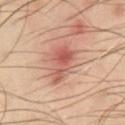lighting: cross-polarized
image: total-body-photography crop, ~15 mm field of view
subject: male, in their mid- to late 40s
image-analysis metrics: internal color variation of about 6 on a 0–10 scale; an automated nevus-likeness rating near 50 out of 100
site: the front of the torso
diameter: about 5 mm